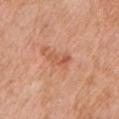- notes — no biopsy performed (imaged during a skin exam)
- lesion size — ~3 mm (longest diameter)
- image — total-body-photography crop, ~15 mm field of view
- patient — male, in their 60s
- tile lighting — white-light illumination
- image-analysis metrics — a lesion area of about 3 mm², a shape eccentricity near 0.9, and two-axis asymmetry of about 0.5; a lesion color around L≈57 a*≈27 b*≈35 in CIELAB and about 9 CIELAB-L* units darker than the surrounding skin
- body site — the left upper arm